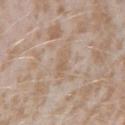body site: the right forearm | lighting: white-light illumination | image: ~15 mm crop, total-body skin-cancer survey | lesion size: ≈3 mm | subject: female, about 25 years old.